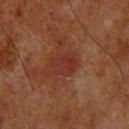Notes:
• notes: catalogued during a skin exam; not biopsied
• site: the head or neck
• subject: male, about 65 years old
• illumination: cross-polarized illumination
• imaging modality: 15 mm crop, total-body photography